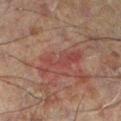No biopsy was performed on this lesion — it was imaged during a full skin examination and was not determined to be concerning.
A close-up tile cropped from a whole-body skin photograph, about 15 mm across.
The subject is a male in their 60s.
From the left lower leg.
Measured at roughly 6 mm in maximum diameter.